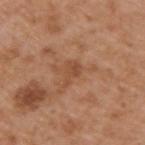Notes:
- acquisition: ~15 mm crop, total-body skin-cancer survey
- anatomic site: the back
- subject: male, aged approximately 65